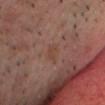Recorded during total-body skin imaging; not selected for excision or biopsy.
From the head or neck.
A region of skin cropped from a whole-body photographic capture, roughly 15 mm wide.
The patient is a male approximately 55 years of age.